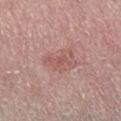Imaged during a routine full-body skin examination; the lesion was not biopsied and no histopathology is available.
A roughly 15 mm field-of-view crop from a total-body skin photograph.
The lesion is located on the leg.
The patient is a male aged around 65.